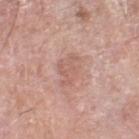biopsy status = imaged on a skin check; not biopsied | subject = male, aged approximately 80 | imaging modality = total-body-photography crop, ~15 mm field of view | illumination = white-light illumination | site = the right thigh.